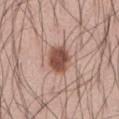– site — the front of the torso
– patient — male, aged 68–72
– imaging modality — ~15 mm crop, total-body skin-cancer survey
– image-analysis metrics — a footprint of about 8.5 mm², an eccentricity of roughly 0.65, and a shape-asymmetry score of about 0.2 (0 = symmetric); a lesion color around L≈50 a*≈22 b*≈27 in CIELAB, a lesion–skin lightness drop of about 15, and a lesion-to-skin contrast of about 10.5 (normalized; higher = more distinct)
– lesion size — ≈3.5 mm
– illumination — white-light